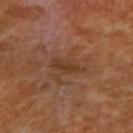follow-up = total-body-photography surveillance lesion; no biopsy | site = the right upper arm | image = 15 mm crop, total-body photography | diameter = ~4 mm (longest diameter) | patient = female, aged around 55.